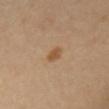Part of a total-body skin-imaging series; this lesion was reviewed on a skin check and was not flagged for biopsy. On the front of the torso. The recorded lesion diameter is about 2.5 mm. Imaged with cross-polarized lighting. A female subject, roughly 55 years of age. Automated tile analysis of the lesion measured a footprint of about 3 mm², a shape eccentricity near 0.8, and two-axis asymmetry of about 0.3. The analysis additionally found a mean CIELAB color near L≈53 a*≈18 b*≈36 and about 9 CIELAB-L* units darker than the surrounding skin. The analysis additionally found a nevus-likeness score of about 85/100 and a detector confidence of about 100 out of 100 that the crop contains a lesion. A 15 mm crop from a total-body photograph taken for skin-cancer surveillance.